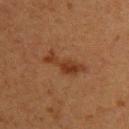The lesion-visualizer software estimated a classifier nevus-likeness of about 50/100 and lesion-presence confidence of about 100/100. The subject is a female aged 38 to 42. This is a cross-polarized tile. A lesion tile, about 15 mm wide, cut from a 3D total-body photograph. The recorded lesion diameter is about 5 mm. On the upper back.